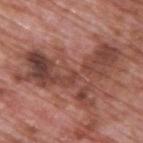Clinical summary: Longest diameter approximately 11.5 mm. A 15 mm crop from a total-body photograph taken for skin-cancer surveillance. A male patient, aged around 70. Imaged with white-light lighting. An algorithmic analysis of the crop reported a border-irregularity index near 9.5/10, a color-variation rating of about 8/10, and radial color variation of about 2.5. The lesion is located on the upper back.